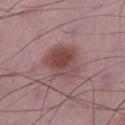  biopsy_status: not biopsied; imaged during a skin examination
  automated_metrics:
    eccentricity: 0.45
    shape_asymmetry: 0.25
    cielab_L: 46
    cielab_a: 22
    cielab_b: 20
    vs_skin_darker_L: 10.0
    vs_skin_contrast_norm: 8.0
    nevus_likeness_0_100: 70
    lesion_detection_confidence_0_100: 100
  site: left lower leg
  lighting: white-light
  image:
    source: total-body photography crop
    field_of_view_mm: 15
  patient:
    sex: male
    age_approx: 50
  lesion_size:
    long_diameter_mm_approx: 4.5